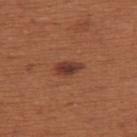Notes:
• biopsy status: total-body-photography surveillance lesion; no biopsy
• lighting: white-light
• image-analysis metrics: radial color variation of about 0.5
• diameter: ≈3 mm
• subject: female, aged 63 to 67
• image: ~15 mm tile from a whole-body skin photo
• anatomic site: the upper back